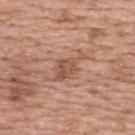Assessment: Part of a total-body skin-imaging series; this lesion was reviewed on a skin check and was not flagged for biopsy. Acquisition and patient details: The subject is a female aged around 60. From the upper back. A region of skin cropped from a whole-body photographic capture, roughly 15 mm wide. The lesion-visualizer software estimated a border-irregularity rating of about 5/10, a within-lesion color-variation index near 2.5/10, and radial color variation of about 1. About 4.5 mm across. Captured under white-light illumination.